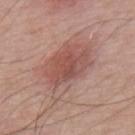acquisition: 15 mm crop, total-body photography
subject: male, in their mid-60s
anatomic site: the upper back
lighting: white-light illumination
automated lesion analysis: a lesion area of about 25 mm², a shape eccentricity near 0.8, and a symmetry-axis asymmetry near 0.2; an average lesion color of about L≈53 a*≈22 b*≈24 (CIELAB), roughly 9 lightness units darker than nearby skin, and a normalized lesion–skin contrast near 6.5; a detector confidence of about 100 out of 100 that the crop contains a lesion
lesion diameter: ≈7 mm